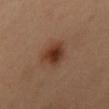* workup · total-body-photography surveillance lesion; no biopsy
* TBP lesion metrics · a border-irregularity index near 2/10, a color-variation rating of about 4.5/10, and peripheral color asymmetry of about 1
* lighting · cross-polarized illumination
* location · the chest
* subject · female, in their mid-30s
* acquisition · 15 mm crop, total-body photography